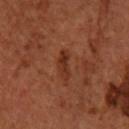{
  "biopsy_status": "not biopsied; imaged during a skin examination",
  "patient": {
    "sex": "female",
    "age_approx": 65
  },
  "site": "right forearm",
  "automated_metrics": {
    "area_mm2_approx": 4.0,
    "eccentricity": 0.95,
    "shape_asymmetry": 0.35,
    "cielab_L": 32,
    "cielab_a": 26,
    "cielab_b": 30,
    "vs_skin_darker_L": 7.0,
    "border_irregularity_0_10": 4.0,
    "color_variation_0_10": 1.5,
    "peripheral_color_asymmetry": 0.0,
    "lesion_detection_confidence_0_100": 100
  },
  "image": {
    "source": "total-body photography crop",
    "field_of_view_mm": 15
  },
  "lighting": "cross-polarized"
}